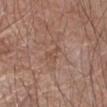This lesion was catalogued during total-body skin photography and was not selected for biopsy.
A close-up tile cropped from a whole-body skin photograph, about 15 mm across.
A male patient, aged 73 to 77.
On the right forearm.